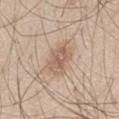biopsy status: total-body-photography surveillance lesion; no biopsy | subject: male, about 60 years old | tile lighting: white-light | image: ~15 mm crop, total-body skin-cancer survey | lesion size: ≈3.5 mm | site: the left thigh.